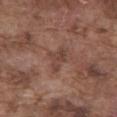<case>
<biopsy_status>not biopsied; imaged during a skin examination</biopsy_status>
<lighting>white-light</lighting>
<image>
  <source>total-body photography crop</source>
  <field_of_view_mm>15</field_of_view_mm>
</image>
<lesion_size>
  <long_diameter_mm_approx>3.0</long_diameter_mm_approx>
</lesion_size>
<site>abdomen</site>
<patient>
  <sex>male</sex>
  <age_approx>75</age_approx>
</patient>
</case>